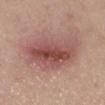biopsy status: no biopsy performed (imaged during a skin exam) | illumination: white-light | anatomic site: the leg | size: about 8.5 mm | image: total-body-photography crop, ~15 mm field of view | image-analysis metrics: an area of roughly 31 mm² and an outline eccentricity of about 0.85 (0 = round, 1 = elongated); a lesion color around L≈51 a*≈23 b*≈23 in CIELAB, roughly 12 lightness units darker than nearby skin, and a normalized border contrast of about 8.5; a border-irregularity rating of about 3/10 and radial color variation of about 2.5; a classifier nevus-likeness of about 95/100 and a lesion-detection confidence of about 100/100 | patient: female, about 40 years old.